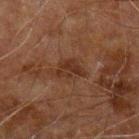Q: Is there a histopathology result?
A: catalogued during a skin exam; not biopsied
Q: Where on the body is the lesion?
A: the left upper arm
Q: What did automated image analysis measure?
A: a footprint of about 6 mm², a shape eccentricity near 0.7, and a shape-asymmetry score of about 0.3 (0 = symmetric); an average lesion color of about L≈24 a*≈16 b*≈23 (CIELAB), about 6 CIELAB-L* units darker than the surrounding skin, and a normalized border contrast of about 6.5; a border-irregularity rating of about 3.5/10, a within-lesion color-variation index near 3/10, and radial color variation of about 1; lesion-presence confidence of about 100/100
Q: Illumination type?
A: cross-polarized
Q: Patient demographics?
A: male, roughly 60 years of age
Q: What kind of image is this?
A: 15 mm crop, total-body photography
Q: How large is the lesion?
A: ~3.5 mm (longest diameter)